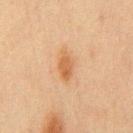Q: Was this lesion biopsied?
A: catalogued during a skin exam; not biopsied
Q: How was the tile lit?
A: cross-polarized
Q: What did automated image analysis measure?
A: a lesion area of about 5 mm² and two-axis asymmetry of about 0.25
Q: Where on the body is the lesion?
A: the front of the torso
Q: What is the imaging modality?
A: total-body-photography crop, ~15 mm field of view
Q: Who is the patient?
A: male, in their mid-40s